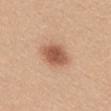workup = total-body-photography surveillance lesion; no biopsy
image source = 15 mm crop, total-body photography
lighting = white-light
subject = female, aged around 30
location = the back
size = about 4.5 mm